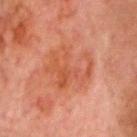<record>
  <biopsy_status>not biopsied; imaged during a skin examination</biopsy_status>
  <site>head or neck</site>
  <automated_metrics>
    <cielab_L>43</cielab_L>
    <cielab_a>24</cielab_a>
    <cielab_b>29</cielab_b>
    <vs_skin_darker_L>6.0</vs_skin_darker_L>
    <border_irregularity_0_10>6.0</border_irregularity_0_10>
    <lesion_detection_confidence_0_100>100</lesion_detection_confidence_0_100>
  </automated_metrics>
  <image>
    <source>total-body photography crop</source>
    <field_of_view_mm>15</field_of_view_mm>
  </image>
  <patient>
    <sex>male</sex>
    <age_approx>80</age_approx>
  </patient>
  <lighting>cross-polarized</lighting>
</record>